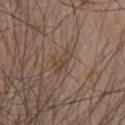size — ≈3.5 mm
anatomic site — the upper back
subject — male, approximately 50 years of age
TBP lesion metrics — an area of roughly 3.5 mm², an outline eccentricity of about 0.9 (0 = round, 1 = elongated), and a shape-asymmetry score of about 0.35 (0 = symmetric); an average lesion color of about L≈43 a*≈14 b*≈25 (CIELAB) and a lesion–skin lightness drop of about 7; a border-irregularity index near 4/10
image — ~15 mm tile from a whole-body skin photo
lighting — white-light illumination